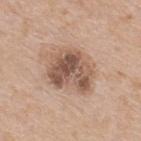Notes:
• workup: imaged on a skin check; not biopsied
• image: 15 mm crop, total-body photography
• site: the upper back
• patient: male, roughly 65 years of age
• automated lesion analysis: an area of roughly 17 mm², an outline eccentricity of about 0.6 (0 = round, 1 = elongated), and two-axis asymmetry of about 0.3; a lesion color around L≈54 a*≈18 b*≈27 in CIELAB and a normalized border contrast of about 9; a classifier nevus-likeness of about 10/100 and a lesion-detection confidence of about 100/100
• lighting: white-light illumination